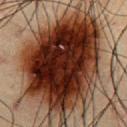follow-up=imaged on a skin check; not biopsied | lighting=cross-polarized illumination | image source=15 mm crop, total-body photography | automated lesion analysis=a footprint of about 80 mm², a shape eccentricity near 0.7, and a symmetry-axis asymmetry near 0.25; a detector confidence of about 100 out of 100 that the crop contains a lesion | lesion size=~13 mm (longest diameter) | patient=male, in their 60s | anatomic site=the chest.